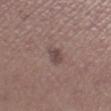Q: Was this lesion biopsied?
A: total-body-photography surveillance lesion; no biopsy
Q: What is the imaging modality?
A: total-body-photography crop, ~15 mm field of view
Q: What is the lesion's diameter?
A: ≈3 mm
Q: What is the anatomic site?
A: the right lower leg
Q: Who is the patient?
A: male, roughly 65 years of age